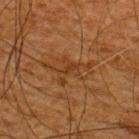biopsy_status: not biopsied; imaged during a skin examination
patient:
  sex: male
  age_approx: 65
image:
  source: total-body photography crop
  field_of_view_mm: 15
site: upper back